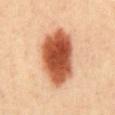This lesion was catalogued during total-body skin photography and was not selected for biopsy.
A roughly 15 mm field-of-view crop from a total-body skin photograph.
The lesion is located on the abdomen.
A male subject roughly 40 years of age.
Longest diameter approximately 7.5 mm.